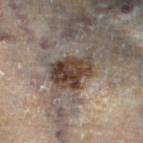{
  "image": {
    "source": "total-body photography crop",
    "field_of_view_mm": 15
  },
  "lesion_size": {
    "long_diameter_mm_approx": 5.0
  },
  "site": "left lower leg",
  "patient": {
    "sex": "female",
    "age_approx": 60
  }
}